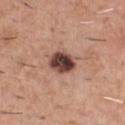follow-up: imaged on a skin check; not biopsied
diameter: about 3.5 mm
image source: ~15 mm tile from a whole-body skin photo
subject: male, aged around 45
body site: the chest